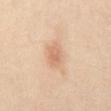Case summary:
– follow-up · total-body-photography surveillance lesion; no biopsy
– patient · male, roughly 50 years of age
– site · the abdomen
– illumination · cross-polarized
– imaging modality · ~15 mm tile from a whole-body skin photo